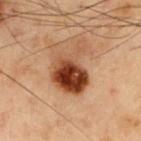| key | value |
|---|---|
| workup | total-body-photography surveillance lesion; no biopsy |
| subject | male, approximately 55 years of age |
| imaging modality | total-body-photography crop, ~15 mm field of view |
| illumination | cross-polarized illumination |
| anatomic site | the chest |